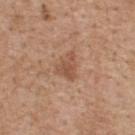Case summary:
• biopsy status — total-body-photography surveillance lesion; no biopsy
• site — the upper back
• image — total-body-photography crop, ~15 mm field of view
• patient — male, approximately 60 years of age
• size — ~3 mm (longest diameter)
• tile lighting — white-light